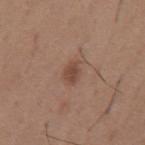follow-up — catalogued during a skin exam; not biopsied | site — the mid back | acquisition — ~15 mm tile from a whole-body skin photo | lesion diameter — ≈3 mm | patient — male, approximately 65 years of age | lighting — white-light.